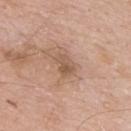Q: How was the tile lit?
A: white-light illumination
Q: What is the anatomic site?
A: the back
Q: What did automated image analysis measure?
A: a normalized border contrast of about 6; radial color variation of about 1.5
Q: Patient demographics?
A: male, roughly 60 years of age
Q: Lesion size?
A: ≈3 mm
Q: What kind of image is this?
A: 15 mm crop, total-body photography